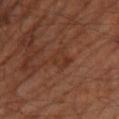location=the leg | patient=male, in their 60s | acquisition=15 mm crop, total-body photography | lighting=cross-polarized | diameter=~4 mm (longest diameter).